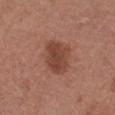Q: Was a biopsy performed?
A: catalogued during a skin exam; not biopsied